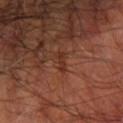Case summary:
- lighting: cross-polarized
- anatomic site: the right forearm
- image source: ~15 mm crop, total-body skin-cancer survey
- subject: male, about 65 years old